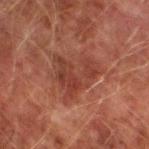<lesion>
<biopsy_status>not biopsied; imaged during a skin examination</biopsy_status>
<patient>
  <sex>male</sex>
  <age_approx>75</age_approx>
</patient>
<lesion_size>
  <long_diameter_mm_approx>5.5</long_diameter_mm_approx>
</lesion_size>
<image>
  <source>total-body photography crop</source>
  <field_of_view_mm>15</field_of_view_mm>
</image>
<lighting>cross-polarized</lighting>
<site>left lower leg</site>
</lesion>